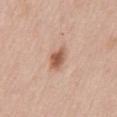Impression: Part of a total-body skin-imaging series; this lesion was reviewed on a skin check and was not flagged for biopsy. Clinical summary: From the mid back. The total-body-photography lesion software estimated border irregularity of about 2.5 on a 0–10 scale, a within-lesion color-variation index near 3/10, and radial color variation of about 1. A female subject in their 40s. A 15 mm crop from a total-body photograph taken for skin-cancer surveillance. The tile uses white-light illumination.